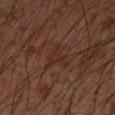Impression:
Recorded during total-body skin imaging; not selected for excision or biopsy.
Context:
About 3.5 mm across. A male patient aged around 55. The lesion is on the arm. Cropped from a whole-body photographic skin survey; the tile spans about 15 mm.